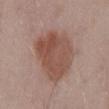Assessment:
This lesion was catalogued during total-body skin photography and was not selected for biopsy.
Context:
An algorithmic analysis of the crop reported a footprint of about 30 mm², an outline eccentricity of about 0.8 (0 = round, 1 = elongated), and a shape-asymmetry score of about 0.2 (0 = symmetric). And it measured roughly 11 lightness units darker than nearby skin and a lesion-to-skin contrast of about 8 (normalized; higher = more distinct). And it measured a border-irregularity rating of about 2.5/10, a within-lesion color-variation index near 4.5/10, and a peripheral color-asymmetry measure near 1.5. And it measured an automated nevus-likeness rating near 90 out of 100 and lesion-presence confidence of about 100/100. Cropped from a whole-body photographic skin survey; the tile spans about 15 mm. The lesion is on the back. Approximately 8 mm at its widest. A male patient, aged approximately 55. Imaged with white-light lighting.